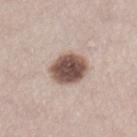notes: no biopsy performed (imaged during a skin exam)
automated metrics: a footprint of about 13 mm², an eccentricity of roughly 0.65, and a shape-asymmetry score of about 0.15 (0 = symmetric); a lesion color around L≈51 a*≈17 b*≈23 in CIELAB and a normalized lesion–skin contrast near 13; border irregularity of about 1.5 on a 0–10 scale, internal color variation of about 5.5 on a 0–10 scale, and radial color variation of about 1.5; a nevus-likeness score of about 95/100 and a detector confidence of about 100 out of 100 that the crop contains a lesion
site: the right lower leg
imaging modality: ~15 mm crop, total-body skin-cancer survey
subject: female, aged 38–42